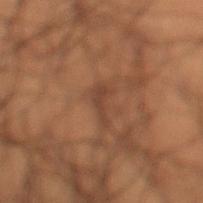No biopsy was performed on this lesion — it was imaged during a full skin examination and was not determined to be concerning.
Captured under cross-polarized illumination.
The subject is a male roughly 50 years of age.
The lesion-visualizer software estimated a footprint of about 3 mm², an eccentricity of roughly 0.85, and a shape-asymmetry score of about 0.6 (0 = symmetric).
A 15 mm close-up extracted from a 3D total-body photography capture.
Measured at roughly 3 mm in maximum diameter.
From the left lower leg.